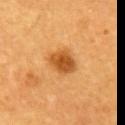On the mid back. A 15 mm crop from a total-body photograph taken for skin-cancer surveillance. The patient is a male about 60 years old.